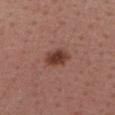Impression:
Imaged during a routine full-body skin examination; the lesion was not biopsied and no histopathology is available.
Context:
Approximately 3.5 mm at its widest. A female patient aged 53–57. An algorithmic analysis of the crop reported a footprint of about 6.5 mm², an outline eccentricity of about 0.75 (0 = round, 1 = elongated), and two-axis asymmetry of about 0.15. It also reported a lesion color around L≈39 a*≈22 b*≈25 in CIELAB, about 12 CIELAB-L* units darker than the surrounding skin, and a normalized lesion–skin contrast near 10. The analysis additionally found border irregularity of about 1.5 on a 0–10 scale, a within-lesion color-variation index near 3.5/10, and radial color variation of about 1. The lesion is located on the chest. The tile uses white-light illumination. This image is a 15 mm lesion crop taken from a total-body photograph.